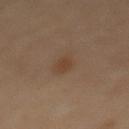Q: Was this lesion biopsied?
A: catalogued during a skin exam; not biopsied
Q: Who is the patient?
A: female, aged around 65
Q: What kind of image is this?
A: ~15 mm tile from a whole-body skin photo
Q: Where on the body is the lesion?
A: the abdomen